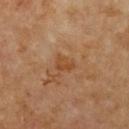biopsy_status: not biopsied; imaged during a skin examination
image:
  source: total-body photography crop
  field_of_view_mm: 15
lighting: cross-polarized
site: chest
lesion_size:
  long_diameter_mm_approx: 2.5
automated_metrics:
  area_mm2_approx: 4.0
  eccentricity: 0.65
  shape_asymmetry: 0.25
  cielab_L: 46
  cielab_a: 22
  cielab_b: 36
  vs_skin_darker_L: 6.0
  border_irregularity_0_10: 2.5
  color_variation_0_10: 2.0
  peripheral_color_asymmetry: 1.0
  nevus_likeness_0_100: 0
  lesion_detection_confidence_0_100: 100
patient:
  sex: female
  age_approx: 65